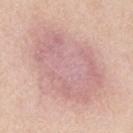Impression:
Captured during whole-body skin photography for melanoma surveillance; the lesion was not biopsied.
Acquisition and patient details:
A male subject, about 60 years old. A 15 mm close-up extracted from a 3D total-body photography capture. The tile uses white-light illumination. The lesion is located on the back. Automated tile analysis of the lesion measured a border-irregularity rating of about 3/10 and peripheral color asymmetry of about 1. It also reported an automated nevus-likeness rating near 0 out of 100 and a detector confidence of about 100 out of 100 that the crop contains a lesion.